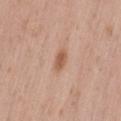No biopsy was performed on this lesion — it was imaged during a full skin examination and was not determined to be concerning. A roughly 15 mm field-of-view crop from a total-body skin photograph. Longest diameter approximately 2.5 mm. Imaged with white-light lighting. A female subject, aged approximately 30. The lesion is located on the lower back.